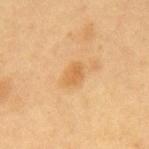| key | value |
|---|---|
| biopsy status | catalogued during a skin exam; not biopsied |
| image | ~15 mm crop, total-body skin-cancer survey |
| lesion diameter | about 3 mm |
| lighting | cross-polarized |
| subject | male, about 55 years old |
| anatomic site | the mid back |